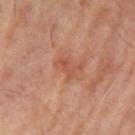Clinical impression: Captured during whole-body skin photography for melanoma surveillance; the lesion was not biopsied. Acquisition and patient details: A male patient, roughly 65 years of age. On the left upper arm. An algorithmic analysis of the crop reported an area of roughly 2 mm², an outline eccentricity of about 0.95 (0 = round, 1 = elongated), and a shape-asymmetry score of about 0.5 (0 = symmetric). And it measured a mean CIELAB color near L≈49 a*≈26 b*≈32, roughly 7 lightness units darker than nearby skin, and a lesion-to-skin contrast of about 5.5 (normalized; higher = more distinct). The software also gave a border-irregularity index near 6.5/10, a color-variation rating of about 0/10, and radial color variation of about 0. Cropped from a total-body skin-imaging series; the visible field is about 15 mm. The tile uses cross-polarized illumination. Longest diameter approximately 2.5 mm.